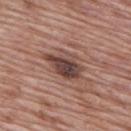Clinical summary: Located on the back. A lesion tile, about 15 mm wide, cut from a 3D total-body photograph. A male patient roughly 70 years of age. This is a white-light tile. Automated tile analysis of the lesion measured an area of roughly 11 mm², a shape eccentricity near 0.75, and a symmetry-axis asymmetry near 0.3. The software also gave a border-irregularity rating of about 3.5/10, internal color variation of about 7 on a 0–10 scale, and a peripheral color-asymmetry measure near 2. The analysis additionally found a classifier nevus-likeness of about 15/100. The recorded lesion diameter is about 5 mm.